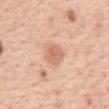The lesion was photographed on a routine skin check and not biopsied; there is no pathology result.
A female patient, roughly 45 years of age.
An algorithmic analysis of the crop reported a color-variation rating of about 3/10. And it measured a nevus-likeness score of about 75/100 and lesion-presence confidence of about 100/100.
A lesion tile, about 15 mm wide, cut from a 3D total-body photograph.
Longest diameter approximately 2.5 mm.
The tile uses white-light illumination.
Located on the front of the torso.